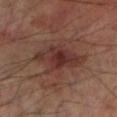Case summary:
• notes · total-body-photography surveillance lesion; no biopsy
• lighting · cross-polarized illumination
• subject · male, aged approximately 70
• diameter · ≈6.5 mm
• body site · the left lower leg
• imaging modality · ~15 mm crop, total-body skin-cancer survey
• TBP lesion metrics · a border-irregularity rating of about 4/10, a color-variation rating of about 4.5/10, and a peripheral color-asymmetry measure near 1.5; a classifier nevus-likeness of about 40/100 and a detector confidence of about 100 out of 100 that the crop contains a lesion